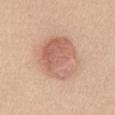notes — no biopsy performed (imaged during a skin exam) | subject — female, aged 28 to 32 | size — about 6.5 mm | anatomic site — the chest | illumination — white-light | image source — total-body-photography crop, ~15 mm field of view.